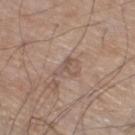Findings:
* biopsy status · total-body-photography surveillance lesion; no biopsy
* body site · the right thigh
* image source · total-body-photography crop, ~15 mm field of view
* patient · male, approximately 70 years of age
* automated metrics · a footprint of about 4.5 mm²